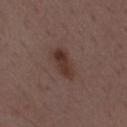  biopsy_status: not biopsied; imaged during a skin examination
  patient:
    sex: male
    age_approx: 50
  automated_metrics:
    area_mm2_approx: 8.0
    shape_asymmetry: 0.15
    cielab_L: 34
    cielab_a: 17
    cielab_b: 22
    vs_skin_contrast_norm: 8.0
    border_irregularity_0_10: 2.5
    peripheral_color_asymmetry: 1.5
    nevus_likeness_0_100: 85
    lesion_detection_confidence_0_100: 100
  site: mid back
  lesion_size:
    long_diameter_mm_approx: 4.5
  image:
    source: total-body photography crop
    field_of_view_mm: 15
  lighting: white-light